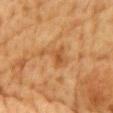{
  "biopsy_status": "not biopsied; imaged during a skin examination",
  "patient": {
    "sex": "male",
    "age_approx": "85+"
  },
  "site": "front of the torso",
  "lighting": "cross-polarized",
  "automated_metrics": {
    "border_irregularity_0_10": 7.5,
    "color_variation_0_10": 3.5,
    "peripheral_color_asymmetry": 1.0,
    "lesion_detection_confidence_0_100": 100
  },
  "lesion_size": {
    "long_diameter_mm_approx": 4.0
  },
  "image": {
    "source": "total-body photography crop",
    "field_of_view_mm": 15
  }
}